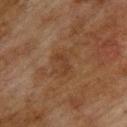Q: Is there a histopathology result?
A: catalogued during a skin exam; not biopsied
Q: Lesion location?
A: the back
Q: How was this image acquired?
A: total-body-photography crop, ~15 mm field of view
Q: How was the tile lit?
A: cross-polarized
Q: Lesion size?
A: ≈3 mm
Q: Who is the patient?
A: male, about 75 years old